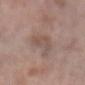Clinical impression:
The lesion was photographed on a routine skin check and not biopsied; there is no pathology result.
Acquisition and patient details:
Longest diameter approximately 3.5 mm. Automated tile analysis of the lesion measured a footprint of about 7 mm² and a shape eccentricity near 0.8. The analysis additionally found a mean CIELAB color near L≈51 a*≈16 b*≈23, about 6 CIELAB-L* units darker than the surrounding skin, and a lesion-to-skin contrast of about 5 (normalized; higher = more distinct). The analysis additionally found lesion-presence confidence of about 100/100. Cropped from a whole-body photographic skin survey; the tile spans about 15 mm. From the left forearm. A female subject, aged 68–72.